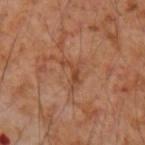Impression:
No biopsy was performed on this lesion — it was imaged during a full skin examination and was not determined to be concerning.
Background:
On the right forearm. Measured at roughly 3.5 mm in maximum diameter. Automated tile analysis of the lesion measured a footprint of about 3.5 mm². And it measured a lesion–skin lightness drop of about 8 and a lesion-to-skin contrast of about 6.5 (normalized; higher = more distinct). It also reported a border-irregularity index near 8/10 and a within-lesion color-variation index near 0/10. The analysis additionally found a lesion-detection confidence of about 100/100. The subject is a male in their mid- to late 50s. A 15 mm close-up tile from a total-body photography series done for melanoma screening. Imaged with cross-polarized lighting.